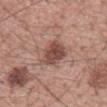Imaged during a routine full-body skin examination; the lesion was not biopsied and no histopathology is available. An algorithmic analysis of the crop reported a lesion area of about 9.5 mm² and an outline eccentricity of about 0.65 (0 = round, 1 = elongated). And it measured a lesion color around L≈48 a*≈21 b*≈24 in CIELAB, about 13 CIELAB-L* units darker than the surrounding skin, and a normalized border contrast of about 9. It also reported a border-irregularity rating of about 2/10, a color-variation rating of about 4/10, and radial color variation of about 1.5. It also reported a lesion-detection confidence of about 100/100. The recorded lesion diameter is about 4 mm. A male patient, aged approximately 55. This is a white-light tile. Cropped from a total-body skin-imaging series; the visible field is about 15 mm. From the back.